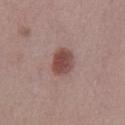biopsy status — total-body-photography surveillance lesion; no biopsy | automated lesion analysis — a lesion color around L≈47 a*≈21 b*≈22 in CIELAB, a lesion–skin lightness drop of about 12, and a normalized border contrast of about 9.5; an automated nevus-likeness rating near 100 out of 100 and a detector confidence of about 100 out of 100 that the crop contains a lesion | image source — ~15 mm tile from a whole-body skin photo | lesion size — ≈3.5 mm | patient — female, aged 38–42 | illumination — white-light illumination | location — the right lower leg.